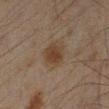The lesion is on the left lower leg. A male subject in their mid-40s. This is a cross-polarized tile. Automated image analysis of the tile measured a lesion area of about 6 mm², an eccentricity of roughly 0.55, and a symmetry-axis asymmetry near 0.2. The analysis additionally found a within-lesion color-variation index near 3/10 and radial color variation of about 1. It also reported an automated nevus-likeness rating near 95 out of 100 and a detector confidence of about 100 out of 100 that the crop contains a lesion. The lesion's longest dimension is about 3 mm. A 15 mm close-up tile from a total-body photography series done for melanoma screening.